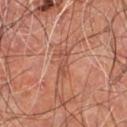Impression:
This lesion was catalogued during total-body skin photography and was not selected for biopsy.
Clinical summary:
A male subject roughly 60 years of age. The lesion is located on the right leg. A lesion tile, about 15 mm wide, cut from a 3D total-body photograph.